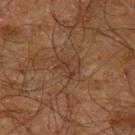Q: Is there a histopathology result?
A: imaged on a skin check; not biopsied
Q: Patient demographics?
A: male, aged approximately 70
Q: What kind of image is this?
A: ~15 mm tile from a whole-body skin photo
Q: What lighting was used for the tile?
A: cross-polarized illumination
Q: How large is the lesion?
A: ~3 mm (longest diameter)
Q: Where on the body is the lesion?
A: the left upper arm